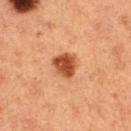biopsy status — imaged on a skin check; not biopsied
tile lighting — cross-polarized illumination
image — ~15 mm tile from a whole-body skin photo
anatomic site — the left thigh
patient — female, about 40 years old
lesion size — about 3 mm